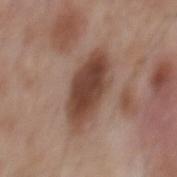The lesion was photographed on a routine skin check and not biopsied; there is no pathology result. The tile uses white-light illumination. A close-up tile cropped from a whole-body skin photograph, about 15 mm across. A male subject, in their mid- to late 50s. About 7 mm across. On the mid back.